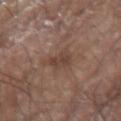Notes:
• biopsy status: catalogued during a skin exam; not biopsied
• tile lighting: white-light
• automated lesion analysis: an average lesion color of about L≈41 a*≈18 b*≈24 (CIELAB), about 8 CIELAB-L* units darker than the surrounding skin, and a normalized lesion–skin contrast near 6.5; a classifier nevus-likeness of about 0/100 and a lesion-detection confidence of about 100/100
• acquisition: ~15 mm crop, total-body skin-cancer survey
• body site: the right forearm
• patient: male, approximately 80 years of age
• size: about 3 mm